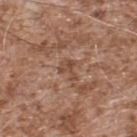Assessment:
Captured during whole-body skin photography for melanoma surveillance; the lesion was not biopsied.
Background:
The lesion's longest dimension is about 2.5 mm. The lesion is located on the upper back. This is a white-light tile. A 15 mm close-up extracted from a 3D total-body photography capture. The patient is a male aged 53–57.